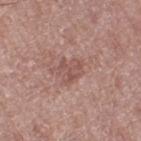Q: Was this lesion biopsied?
A: catalogued during a skin exam; not biopsied
Q: What is the lesion's diameter?
A: ~3 mm (longest diameter)
Q: What kind of image is this?
A: ~15 mm tile from a whole-body skin photo
Q: Lesion location?
A: the left thigh
Q: Who is the patient?
A: female, in their 80s
Q: How was the tile lit?
A: white-light illumination